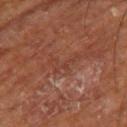Q: Who is the patient?
A: male, in their mid- to late 60s
Q: How was the tile lit?
A: cross-polarized
Q: Lesion size?
A: ~3.5 mm (longest diameter)
Q: What is the anatomic site?
A: the right thigh
Q: What did automated image analysis measure?
A: a footprint of about 5 mm², a shape eccentricity near 0.8, and a symmetry-axis asymmetry near 0.7; a lesion color around L≈38 a*≈23 b*≈28 in CIELAB, about 5 CIELAB-L* units darker than the surrounding skin, and a lesion-to-skin contrast of about 4.5 (normalized; higher = more distinct); a within-lesion color-variation index near 0/10 and a peripheral color-asymmetry measure near 0
Q: What kind of image is this?
A: total-body-photography crop, ~15 mm field of view